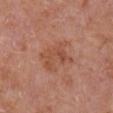Q: Was this lesion biopsied?
A: catalogued during a skin exam; not biopsied
Q: Lesion size?
A: about 4.5 mm
Q: What kind of image is this?
A: ~15 mm crop, total-body skin-cancer survey
Q: Patient demographics?
A: male, in their mid- to late 60s
Q: Lesion location?
A: the right upper arm
Q: Illumination type?
A: white-light
Q: What did automated image analysis measure?
A: an area of roughly 11 mm², an outline eccentricity of about 0.65 (0 = round, 1 = elongated), and a shape-asymmetry score of about 0.25 (0 = symmetric); a mean CIELAB color near L≈51 a*≈25 b*≈31, roughly 7 lightness units darker than nearby skin, and a lesion-to-skin contrast of about 5.5 (normalized; higher = more distinct); a border-irregularity rating of about 4/10 and a peripheral color-asymmetry measure near 1.5